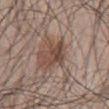Q: What are the patient's age and sex?
A: male, aged approximately 50
Q: What kind of image is this?
A: 15 mm crop, total-body photography
Q: Illumination type?
A: white-light illumination
Q: Where on the body is the lesion?
A: the abdomen
Q: Automated lesion metrics?
A: a footprint of about 7 mm² and a shape-asymmetry score of about 0.35 (0 = symmetric); a border-irregularity index near 4/10, a within-lesion color-variation index near 5.5/10, and a peripheral color-asymmetry measure near 2; lesion-presence confidence of about 95/100